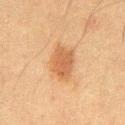Assessment: Recorded during total-body skin imaging; not selected for excision or biopsy. Context: On the abdomen. A male subject, aged around 50. Cropped from a whole-body photographic skin survey; the tile spans about 15 mm.